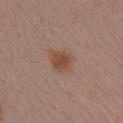Findings:
* follow-up — imaged on a skin check; not biopsied
* patient — female, in their mid- to late 50s
* TBP lesion metrics — a footprint of about 7 mm², a shape eccentricity near 0.65, and a shape-asymmetry score of about 0.15 (0 = symmetric); a detector confidence of about 100 out of 100 that the crop contains a lesion
* image source — 15 mm crop, total-body photography
* body site — the arm
* diameter — ≈3.5 mm